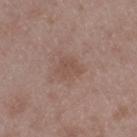Part of a total-body skin-imaging series; this lesion was reviewed on a skin check and was not flagged for biopsy. A roughly 15 mm field-of-view crop from a total-body skin photograph. Automated image analysis of the tile measured an area of roughly 6 mm² and an eccentricity of roughly 0.45. And it measured an automated nevus-likeness rating near 0 out of 100 and lesion-presence confidence of about 100/100. The subject is a male approximately 50 years of age. The lesion is located on the left thigh. Approximately 3 mm at its widest. Imaged with white-light lighting.